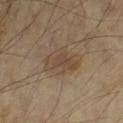biopsy_status: not biopsied; imaged during a skin examination
image:
  source: total-body photography crop
  field_of_view_mm: 15
site: right upper arm
patient:
  sex: male
  age_approx: 60